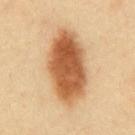Clinical impression:
This lesion was catalogued during total-body skin photography and was not selected for biopsy.
Background:
The tile uses cross-polarized illumination. An algorithmic analysis of the crop reported a lesion area of about 31 mm² and an eccentricity of roughly 0.85. It also reported a lesion–skin lightness drop of about 18 and a lesion-to-skin contrast of about 11.5 (normalized; higher = more distinct). The analysis additionally found a color-variation rating of about 6.5/10 and radial color variation of about 2. This image is a 15 mm lesion crop taken from a total-body photograph. Located on the front of the torso. The patient is a male in their 40s.